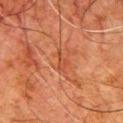| feature | finding |
|---|---|
| follow-up | no biopsy performed (imaged during a skin exam) |
| body site | the chest |
| image-analysis metrics | an area of roughly 3 mm²; a mean CIELAB color near L≈39 a*≈25 b*≈32 and a normalized lesion–skin contrast near 5; internal color variation of about 1.5 on a 0–10 scale and peripheral color asymmetry of about 0.5 |
| patient | male, in their 80s |
| tile lighting | cross-polarized |
| lesion size | about 2.5 mm |
| imaging modality | ~15 mm crop, total-body skin-cancer survey |